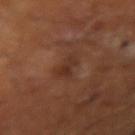Clinical impression: Captured during whole-body skin photography for melanoma surveillance; the lesion was not biopsied. Context: A male patient, roughly 65 years of age. Captured under cross-polarized illumination. The total-body-photography lesion software estimated an area of roughly 5.5 mm², an eccentricity of roughly 0.75, and a shape-asymmetry score of about 0.4 (0 = symmetric). And it measured about 6 CIELAB-L* units darker than the surrounding skin and a normalized lesion–skin contrast near 6. It also reported a nevus-likeness score of about 0/100. Measured at roughly 3.5 mm in maximum diameter. A close-up tile cropped from a whole-body skin photograph, about 15 mm across.